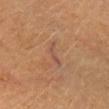follow-up=no biopsy performed (imaged during a skin exam)
body site=the head or neck
illumination=cross-polarized
acquisition=~15 mm tile from a whole-body skin photo
automated metrics=a lesion area of about 4 mm², a shape eccentricity near 0.95, and a symmetry-axis asymmetry near 0.5; an automated nevus-likeness rating near 0 out of 100
patient=female, aged around 55
lesion size=about 4 mm